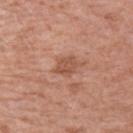Assessment: No biopsy was performed on this lesion — it was imaged during a full skin examination and was not determined to be concerning. Image and clinical context: Approximately 2.5 mm at its widest. The total-body-photography lesion software estimated a border-irregularity rating of about 2.5/10, a within-lesion color-variation index near 2/10, and peripheral color asymmetry of about 0.5. And it measured a nevus-likeness score of about 0/100 and lesion-presence confidence of about 100/100. The lesion is located on the left upper arm. Cropped from a whole-body photographic skin survey; the tile spans about 15 mm. A female patient, aged around 70.